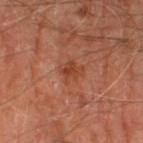Notes:
- follow-up: catalogued during a skin exam; not biopsied
- lesion size: about 2.5 mm
- patient: male, aged 68 to 72
- image source: 15 mm crop, total-body photography
- site: the left thigh